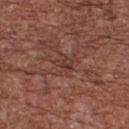This lesion was catalogued during total-body skin photography and was not selected for biopsy.
This is a white-light tile.
The total-body-photography lesion software estimated an area of roughly 3 mm² and a shape eccentricity near 0.8. The software also gave a detector confidence of about 75 out of 100 that the crop contains a lesion.
A 15 mm close-up extracted from a 3D total-body photography capture.
Located on the upper back.
The patient is a male aged approximately 75.
The lesion's longest dimension is about 2.5 mm.